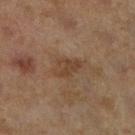Imaged during a routine full-body skin examination; the lesion was not biopsied and no histopathology is available.
The patient is a female aged 58–62.
On the right lower leg.
A 15 mm crop from a total-body photograph taken for skin-cancer surveillance.
This is a cross-polarized tile.